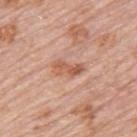workup = catalogued during a skin exam; not biopsied.